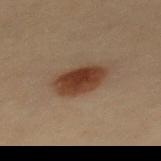<lesion>
<site>mid back</site>
<image>
  <source>total-body photography crop</source>
  <field_of_view_mm>15</field_of_view_mm>
</image>
<patient>
  <sex>female</sex>
  <age_approx>30</age_approx>
</patient>
</lesion>